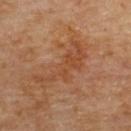Q: Was a biopsy performed?
A: no biopsy performed (imaged during a skin exam)